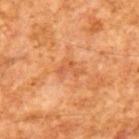This lesion was catalogued during total-body skin photography and was not selected for biopsy. This is a cross-polarized tile. A male patient aged 63 to 67. Approximately 3 mm at its widest. A lesion tile, about 15 mm wide, cut from a 3D total-body photograph.